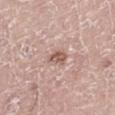{"biopsy_status": "not biopsied; imaged during a skin examination", "patient": {"sex": "male", "age_approx": 70}, "site": "left leg", "lighting": "white-light", "image": {"source": "total-body photography crop", "field_of_view_mm": 15}, "lesion_size": {"long_diameter_mm_approx": 3.0}}